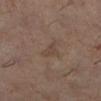Assessment:
Captured during whole-body skin photography for melanoma surveillance; the lesion was not biopsied.
Image and clinical context:
Imaged with cross-polarized lighting. A lesion tile, about 15 mm wide, cut from a 3D total-body photograph. On the left lower leg. A female subject, aged 58–62. Automated image analysis of the tile measured an average lesion color of about L≈43 a*≈15 b*≈24 (CIELAB), roughly 6 lightness units darker than nearby skin, and a normalized lesion–skin contrast near 5. It also reported border irregularity of about 5.5 on a 0–10 scale, internal color variation of about 1.5 on a 0–10 scale, and a peripheral color-asymmetry measure near 0.5.